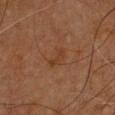<tbp_lesion>
<biopsy_status>not biopsied; imaged during a skin examination</biopsy_status>
<automated_metrics>
  <area_mm2_approx>2.5</area_mm2_approx>
  <eccentricity>0.9</eccentricity>
  <shape_asymmetry>0.45</shape_asymmetry>
  <cielab_L>31</cielab_L>
  <cielab_a>18</cielab_a>
  <cielab_b>28</cielab_b>
  <vs_skin_darker_L>4.0</vs_skin_darker_L>
  <vs_skin_contrast_norm>5.5</vs_skin_contrast_norm>
  <color_variation_0_10>0.0</color_variation_0_10>
  <nevus_likeness_0_100>0</nevus_likeness_0_100>
  <lesion_detection_confidence_0_100>100</lesion_detection_confidence_0_100>
</automated_metrics>
<patient>
  <sex>male</sex>
  <age_approx>65</age_approx>
</patient>
<lesion_size>
  <long_diameter_mm_approx>3.0</long_diameter_mm_approx>
</lesion_size>
<image>
  <source>total-body photography crop</source>
  <field_of_view_mm>15</field_of_view_mm>
</image>
<site>chest</site>
<lighting>cross-polarized</lighting>
</tbp_lesion>